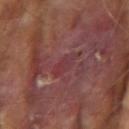The lesion was photographed on a routine skin check and not biopsied; there is no pathology result. A 15 mm close-up tile from a total-body photography series done for melanoma screening. Imaged with cross-polarized lighting. The lesion's longest dimension is about 3.5 mm. A male patient aged around 70. Located on the right upper arm.